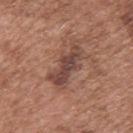<case>
<biopsy_status>not biopsied; imaged during a skin examination</biopsy_status>
<automated_metrics>
  <area_mm2_approx>12.0</area_mm2_approx>
  <shape_asymmetry>0.35</shape_asymmetry>
  <vs_skin_contrast_norm>8.0</vs_skin_contrast_norm>
</automated_metrics>
<lighting>white-light</lighting>
<patient>
  <sex>male</sex>
  <age_approx>75</age_approx>
</patient>
<image>
  <source>total-body photography crop</source>
  <field_of_view_mm>15</field_of_view_mm>
</image>
<site>upper back</site>
</case>